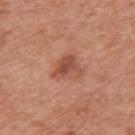Recorded during total-body skin imaging; not selected for excision or biopsy. This is a white-light tile. The lesion's longest dimension is about 3.5 mm. An algorithmic analysis of the crop reported a lesion area of about 5.5 mm² and an outline eccentricity of about 0.65 (0 = round, 1 = elongated). And it measured a lesion–skin lightness drop of about 10 and a normalized lesion–skin contrast near 7.5. It also reported a classifier nevus-likeness of about 45/100 and a detector confidence of about 100 out of 100 that the crop contains a lesion. The patient is a male aged 68 to 72. A close-up tile cropped from a whole-body skin photograph, about 15 mm across. From the upper back.